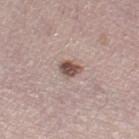notes = no biopsy performed (imaged during a skin exam); subject = female, aged 48 to 52; lighting = white-light; imaging modality = 15 mm crop, total-body photography; location = the right thigh.